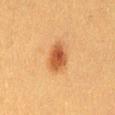workup = total-body-photography surveillance lesion; no biopsy | lighting = cross-polarized illumination | size = about 3.5 mm | image = 15 mm crop, total-body photography | image-analysis metrics = an area of roughly 7 mm² and a shape eccentricity near 0.7; an average lesion color of about L≈45 a*≈24 b*≈36 (CIELAB) and about 12 CIELAB-L* units darker than the surrounding skin; a classifier nevus-likeness of about 100/100 and a lesion-detection confidence of about 100/100 | location = the abdomen | subject = female, roughly 40 years of age.